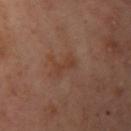• notes — total-body-photography surveillance lesion; no biopsy
• image — ~15 mm tile from a whole-body skin photo
• lighting — cross-polarized
• body site — the left upper arm
• lesion diameter — ≈3 mm
• subject — female, aged around 55